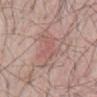Notes:
– biopsy status: catalogued during a skin exam; not biopsied
– image: ~15 mm crop, total-body skin-cancer survey
– body site: the mid back
– lighting: white-light illumination
– patient: male, roughly 65 years of age